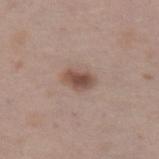follow-up: no biopsy performed (imaged during a skin exam)
location: the abdomen
lighting: white-light
TBP lesion metrics: an area of roughly 6 mm², an outline eccentricity of about 0.8 (0 = round, 1 = elongated), and two-axis asymmetry of about 0.2
size: ~3.5 mm (longest diameter)
patient: female, aged 38–42
imaging modality: total-body-photography crop, ~15 mm field of view